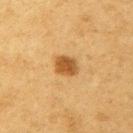<tbp_lesion>
<site>left upper arm</site>
<image>
  <source>total-body photography crop</source>
  <field_of_view_mm>15</field_of_view_mm>
</image>
<patient>
  <sex>male</sex>
  <age_approx>55</age_approx>
</patient>
<lighting>cross-polarized</lighting>
</tbp_lesion>